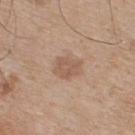biopsy status: catalogued during a skin exam; not biopsied | patient: male, roughly 75 years of age | site: the upper back | acquisition: 15 mm crop, total-body photography | diameter: ≈3.5 mm.